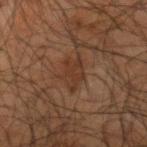This lesion was catalogued during total-body skin photography and was not selected for biopsy. The lesion-visualizer software estimated a lesion color around L≈28 a*≈16 b*≈24 in CIELAB, about 5 CIELAB-L* units darker than the surrounding skin, and a normalized lesion–skin contrast near 6. This is a cross-polarized tile. Cropped from a total-body skin-imaging series; the visible field is about 15 mm. A male patient, in their mid- to late 60s. On the right upper arm.